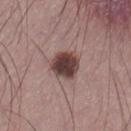notes = no biopsy performed (imaged during a skin exam) | imaging modality = ~15 mm crop, total-body skin-cancer survey | tile lighting = white-light illumination | anatomic site = the right lower leg | subject = male, aged 33–37 | diameter = ≈4 mm.